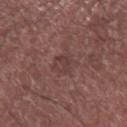workup: total-body-photography surveillance lesion; no biopsy
image: 15 mm crop, total-body photography
body site: the right forearm
image-analysis metrics: a mean CIELAB color near L≈39 a*≈20 b*≈20, a lesion–skin lightness drop of about 5, and a normalized border contrast of about 4.5; a border-irregularity rating of about 4/10 and a within-lesion color-variation index near 2/10; an automated nevus-likeness rating near 0 out of 100 and a detector confidence of about 90 out of 100 that the crop contains a lesion
patient: male, approximately 70 years of age